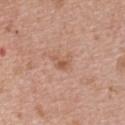No biopsy was performed on this lesion — it was imaged during a full skin examination and was not determined to be concerning.